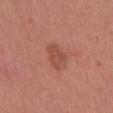image source — ~15 mm tile from a whole-body skin photo
image-analysis metrics — a lesion area of about 5.5 mm², an eccentricity of roughly 0.85, and a shape-asymmetry score of about 0.3 (0 = symmetric); a lesion–skin lightness drop of about 8 and a lesion-to-skin contrast of about 6 (normalized; higher = more distinct); a classifier nevus-likeness of about 5/100
diameter — about 3.5 mm
illumination — white-light
patient — male, in their mid-40s
anatomic site — the upper back